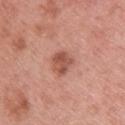Impression:
Part of a total-body skin-imaging series; this lesion was reviewed on a skin check and was not flagged for biopsy.
Context:
A roughly 15 mm field-of-view crop from a total-body skin photograph. From the upper back. The lesion's longest dimension is about 3 mm. The tile uses white-light illumination. A male patient roughly 60 years of age.